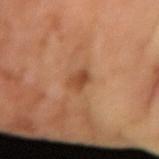The lesion was tiled from a total-body skin photograph and was not biopsied. Measured at roughly 2.5 mm in maximum diameter. A 15 mm close-up extracted from a 3D total-body photography capture. The subject is a male aged around 65.